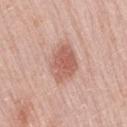<record>
<biopsy_status>not biopsied; imaged during a skin examination</biopsy_status>
<site>lower back</site>
<image>
  <source>total-body photography crop</source>
  <field_of_view_mm>15</field_of_view_mm>
</image>
<lesion_size>
  <long_diameter_mm_approx>4.5</long_diameter_mm_approx>
</lesion_size>
<lighting>white-light</lighting>
<automated_metrics>
  <border_irregularity_0_10>2.0</border_irregularity_0_10>
  <color_variation_0_10>3.5</color_variation_0_10>
  <peripheral_color_asymmetry>1.0</peripheral_color_asymmetry>
</automated_metrics>
<patient>
  <sex>female</sex>
  <age_approx>60</age_approx>
</patient>
</record>